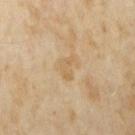Clinical impression:
The lesion was photographed on a routine skin check and not biopsied; there is no pathology result.
Clinical summary:
On the arm. Cropped from a total-body skin-imaging series; the visible field is about 15 mm. The tile uses cross-polarized illumination. The patient is a female in their mid-30s.